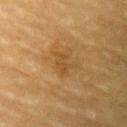notes: total-body-photography surveillance lesion; no biopsy
illumination: cross-polarized
image source: ~15 mm tile from a whole-body skin photo
patient: male, aged approximately 85 or older
anatomic site: the left upper arm
lesion diameter: about 3 mm